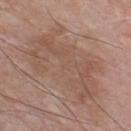This lesion was catalogued during total-body skin photography and was not selected for biopsy. On the chest. About 10 mm across. Imaged with white-light lighting. Cropped from a total-body skin-imaging series; the visible field is about 15 mm. The subject is a male approximately 80 years of age.